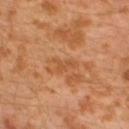Findings:
- workup — total-body-photography surveillance lesion; no biopsy
- subject — male, in their 30s
- tile lighting — cross-polarized illumination
- body site — the left lower leg
- automated lesion analysis — a footprint of about 3 mm² and an eccentricity of roughly 0.85; an average lesion color of about L≈51 a*≈24 b*≈39 (CIELAB) and a normalized lesion–skin contrast near 5; border irregularity of about 5.5 on a 0–10 scale, a within-lesion color-variation index near 0/10, and a peripheral color-asymmetry measure near 0
- acquisition — total-body-photography crop, ~15 mm field of view
- lesion size — ≈3 mm